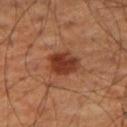follow-up — total-body-photography surveillance lesion; no biopsy
image source — ~15 mm crop, total-body skin-cancer survey
lighting — cross-polarized illumination
body site — the leg
lesion diameter — about 4 mm
subject — male, roughly 70 years of age